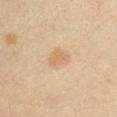follow-up: imaged on a skin check; not biopsied | imaging modality: ~15 mm tile from a whole-body skin photo | diameter: about 3 mm | body site: the front of the torso | patient: male, in their 60s | tile lighting: cross-polarized.